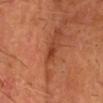<tbp_lesion>
<image>
  <source>total-body photography crop</source>
  <field_of_view_mm>15</field_of_view_mm>
</image>
<lighting>cross-polarized</lighting>
<site>head or neck</site>
<automated_metrics>
  <area_mm2_approx>2.5</area_mm2_approx>
  <eccentricity>0.9</eccentricity>
  <shape_asymmetry>0.35</shape_asymmetry>
  <cielab_L>42</cielab_L>
  <cielab_a>30</cielab_a>
  <cielab_b>37</cielab_b>
  <vs_skin_darker_L>9.0</vs_skin_darker_L>
  <vs_skin_contrast_norm>7.0</vs_skin_contrast_norm>
  <border_irregularity_0_10>4.0</border_irregularity_0_10>
  <peripheral_color_asymmetry>0.0</peripheral_color_asymmetry>
  <nevus_likeness_0_100>0</nevus_likeness_0_100>
  <lesion_detection_confidence_0_100>85</lesion_detection_confidence_0_100>
</automated_metrics>
<patient>
  <sex>male</sex>
  <age_approx>65</age_approx>
</patient>
</tbp_lesion>